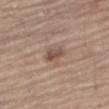Part of a total-body skin-imaging series; this lesion was reviewed on a skin check and was not flagged for biopsy. Longest diameter approximately 2.5 mm. A 15 mm crop from a total-body photograph taken for skin-cancer surveillance. Captured under white-light illumination. A male subject aged around 65. The lesion is located on the leg.